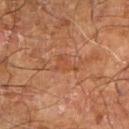The lesion was tiled from a total-body skin photograph and was not biopsied. A region of skin cropped from a whole-body photographic capture, roughly 15 mm wide. On the right forearm. A male subject, aged 68–72.